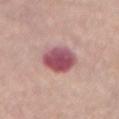{
  "biopsy_status": "not biopsied; imaged during a skin examination",
  "automated_metrics": {
    "area_mm2_approx": 12.0,
    "eccentricity": 0.7,
    "shape_asymmetry": 0.15,
    "cielab_L": 52,
    "cielab_a": 28,
    "cielab_b": 17,
    "vs_skin_darker_L": 16.0,
    "vs_skin_contrast_norm": 11.5,
    "border_irregularity_0_10": 1.5,
    "color_variation_0_10": 7.0,
    "peripheral_color_asymmetry": 2.0
  },
  "lesion_size": {
    "long_diameter_mm_approx": 4.5
  },
  "patient": {
    "sex": "female",
    "age_approx": 65
  },
  "site": "abdomen",
  "image": {
    "source": "total-body photography crop",
    "field_of_view_mm": 15
  }
}